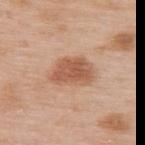The patient is a female aged approximately 50.
A 15 mm close-up tile from a total-body photography series done for melanoma screening.
The lesion is on the upper back.
Captured under white-light illumination.
Approximately 5 mm at its widest.